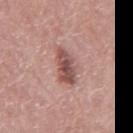{
  "biopsy_status": "not biopsied; imaged during a skin examination",
  "automated_metrics": {
    "area_mm2_approx": 8.0,
    "eccentricity": 0.8,
    "shape_asymmetry": 0.25
  },
  "site": "mid back",
  "patient": {
    "sex": "male",
    "age_approx": 70
  },
  "lesion_size": {
    "long_diameter_mm_approx": 4.0
  },
  "lighting": "white-light",
  "image": {
    "source": "total-body photography crop",
    "field_of_view_mm": 15
  }
}